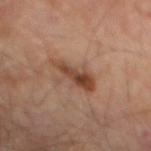Assessment: The lesion was tiled from a total-body skin photograph and was not biopsied. Clinical summary: This is a cross-polarized tile. A lesion tile, about 15 mm wide, cut from a 3D total-body photograph. A male subject aged 68 to 72. The total-body-photography lesion software estimated an area of roughly 8 mm², a shape eccentricity near 0.9, and a symmetry-axis asymmetry near 0.4. It also reported a border-irregularity rating of about 5/10, a within-lesion color-variation index near 5.5/10, and a peripheral color-asymmetry measure near 2. On the mid back.